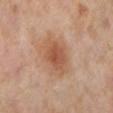Notes:
– notes — total-body-photography surveillance lesion; no biopsy
– subject — female, approximately 55 years of age
– site — the left lower leg
– lesion size — about 4 mm
– tile lighting — cross-polarized
– acquisition — 15 mm crop, total-body photography